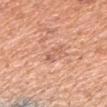| feature | finding |
|---|---|
| notes | no biopsy performed (imaged during a skin exam) |
| size | ≈3 mm |
| anatomic site | the left upper arm |
| automated lesion analysis | an area of roughly 3.5 mm², an eccentricity of roughly 0.85, and a shape-asymmetry score of about 0.35 (0 = symmetric); a mean CIELAB color near L≈62 a*≈24 b*≈32 |
| image source | ~15 mm tile from a whole-body skin photo |
| lighting | white-light illumination |
| patient | male, roughly 65 years of age |